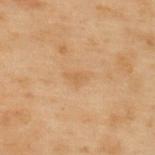Q: How large is the lesion?
A: ≈2.5 mm
Q: What is the imaging modality?
A: ~15 mm crop, total-body skin-cancer survey
Q: What are the patient's age and sex?
A: male, aged approximately 55
Q: Lesion location?
A: the upper back
Q: What lighting was used for the tile?
A: cross-polarized